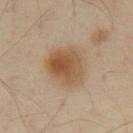Acquisition and patient details: Longest diameter approximately 4.5 mm. The lesion is on the abdomen. A region of skin cropped from a whole-body photographic capture, roughly 15 mm wide. Captured under cross-polarized illumination. The subject is a male aged 53 to 57.